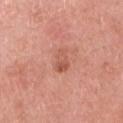This lesion was catalogued during total-body skin photography and was not selected for biopsy. Cropped from a total-body skin-imaging series; the visible field is about 15 mm. The patient is a female aged 68 to 72. The lesion is located on the right upper arm. Approximately 3 mm at its widest. Imaged with white-light lighting.